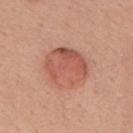Case summary:
* image-analysis metrics · a lesion area of about 18 mm² and a shape-asymmetry score of about 0.2 (0 = symmetric); a lesion color around L≈56 a*≈27 b*≈29 in CIELAB, a lesion–skin lightness drop of about 9, and a lesion-to-skin contrast of about 6.5 (normalized; higher = more distinct); a border-irregularity index near 2.5/10, internal color variation of about 5 on a 0–10 scale, and a peripheral color-asymmetry measure near 1.5
* lesion diameter · about 5 mm
* image source · ~15 mm crop, total-body skin-cancer survey
* site · the mid back
* patient · female, aged around 55
* illumination · white-light